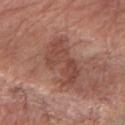Clinical impression:
No biopsy was performed on this lesion — it was imaged during a full skin examination and was not determined to be concerning.
Clinical summary:
This is a white-light tile. Automated tile analysis of the lesion measured a footprint of about 14 mm² and a shape-asymmetry score of about 0.45 (0 = symmetric). It also reported a border-irregularity index near 7/10 and a within-lesion color-variation index near 3/10. It also reported a nevus-likeness score of about 0/100 and a detector confidence of about 95 out of 100 that the crop contains a lesion. A 15 mm close-up extracted from a 3D total-body photography capture. From the right forearm. A female patient, aged around 75. Measured at roughly 6 mm in maximum diameter.